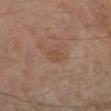The lesion was tiled from a total-body skin photograph and was not biopsied. Automated image analysis of the tile measured an area of roughly 4 mm² and a shape eccentricity near 0.7. And it measured a border-irregularity index near 2.5/10, a color-variation rating of about 1.5/10, and peripheral color asymmetry of about 0.5. And it measured an automated nevus-likeness rating near 0 out of 100 and a lesion-detection confidence of about 100/100. Located on the left lower leg. Captured under cross-polarized illumination. A close-up tile cropped from a whole-body skin photograph, about 15 mm across. A male subject, about 60 years old.